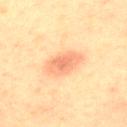Recorded during total-body skin imaging; not selected for excision or biopsy.
Longest diameter approximately 5 mm.
Captured under cross-polarized illumination.
The lesion-visualizer software estimated a lesion area of about 11 mm², an outline eccentricity of about 0.8 (0 = round, 1 = elongated), and a shape-asymmetry score of about 0.15 (0 = symmetric). It also reported a nevus-likeness score of about 85/100 and lesion-presence confidence of about 100/100.
A male patient, aged 63 to 67.
A lesion tile, about 15 mm wide, cut from a 3D total-body photograph.
Located on the chest.